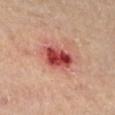The lesion is on the left lower leg. A male patient, aged 63 to 67. Cropped from a total-body skin-imaging series; the visible field is about 15 mm.